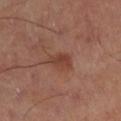Recorded during total-body skin imaging; not selected for excision or biopsy. The tile uses cross-polarized illumination. A lesion tile, about 15 mm wide, cut from a 3D total-body photograph. Located on the right thigh. The lesion-visualizer software estimated a lesion color around L≈41 a*≈24 b*≈28 in CIELAB and a lesion–skin lightness drop of about 8. And it measured border irregularity of about 2.5 on a 0–10 scale, a color-variation rating of about 3/10, and a peripheral color-asymmetry measure near 1. It also reported a classifier nevus-likeness of about 20/100 and a detector confidence of about 100 out of 100 that the crop contains a lesion.